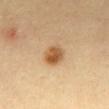The lesion was photographed on a routine skin check and not biopsied; there is no pathology result.
Located on the mid back.
A female subject, aged 38–42.
Cropped from a total-body skin-imaging series; the visible field is about 15 mm.
Longest diameter approximately 3 mm.